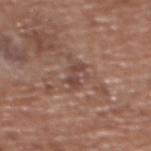notes = catalogued during a skin exam; not biopsied | imaging modality = ~15 mm tile from a whole-body skin photo | site = the back | patient = female, aged 73–77.